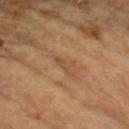• follow-up · no biopsy performed (imaged during a skin exam)
• patient · female, aged around 60
• TBP lesion metrics · an area of roughly 2.5 mm², an eccentricity of roughly 0.9, and two-axis asymmetry of about 0.5; an average lesion color of about L≈50 a*≈22 b*≈35 (CIELAB), about 6 CIELAB-L* units darker than the surrounding skin, and a normalized border contrast of about 5; a nevus-likeness score of about 0/100 and lesion-presence confidence of about 95/100
• imaging modality · ~15 mm tile from a whole-body skin photo
• anatomic site · the left forearm
• lesion size · ≈2.5 mm